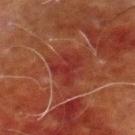Impression: Recorded during total-body skin imaging; not selected for excision or biopsy. Background: Captured under cross-polarized illumination. A 15 mm close-up extracted from a 3D total-body photography capture. The lesion is on the leg. The patient is a male roughly 70 years of age. The lesion's longest dimension is about 4 mm.